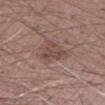{"biopsy_status": "not biopsied; imaged during a skin examination", "site": "left forearm", "lesion_size": {"long_diameter_mm_approx": 4.0}, "patient": {"sex": "male", "age_approx": 50}, "automated_metrics": {"cielab_L": 46, "cielab_a": 18, "cielab_b": 22, "vs_skin_darker_L": 7.0, "vs_skin_contrast_norm": 6.0, "nevus_likeness_0_100": 10, "lesion_detection_confidence_0_100": 100}, "lighting": "white-light", "image": {"source": "total-body photography crop", "field_of_view_mm": 15}}